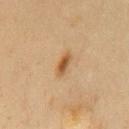Findings:
• notes · imaged on a skin check; not biopsied
• location · the chest
• patient · male, aged 58–62
• illumination · cross-polarized illumination
• acquisition · ~15 mm crop, total-body skin-cancer survey
• diameter · ≈3.5 mm